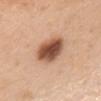| field | value |
|---|---|
| workup | total-body-photography surveillance lesion; no biopsy |
| site | the front of the torso |
| patient | female, about 55 years old |
| imaging modality | ~15 mm tile from a whole-body skin photo |